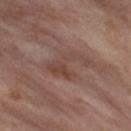The lesion was photographed on a routine skin check and not biopsied; there is no pathology result. The lesion is on the left thigh. A female subject aged around 55. Cropped from a whole-body photographic skin survey; the tile spans about 15 mm.